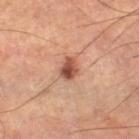Captured during whole-body skin photography for melanoma surveillance; the lesion was not biopsied.
Measured at roughly 2.5 mm in maximum diameter.
A region of skin cropped from a whole-body photographic capture, roughly 15 mm wide.
Located on the leg.
A male subject aged approximately 65.
This is a cross-polarized tile.